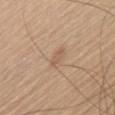Impression:
Recorded during total-body skin imaging; not selected for excision or biopsy.
Image and clinical context:
A lesion tile, about 15 mm wide, cut from a 3D total-body photograph. Approximately 2.5 mm at its widest. A male subject aged 68–72. Captured under white-light illumination. The total-body-photography lesion software estimated a border-irregularity index near 2.5/10 and a color-variation rating of about 0.5/10. On the chest.